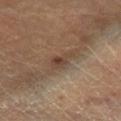No biopsy was performed on this lesion — it was imaged during a full skin examination and was not determined to be concerning. Captured under cross-polarized illumination. On the right lower leg. A male patient in their mid-50s. Cropped from a total-body skin-imaging series; the visible field is about 15 mm.